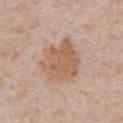{"lighting": "white-light", "lesion_size": {"long_diameter_mm_approx": 5.0}, "image": {"source": "total-body photography crop", "field_of_view_mm": 15}, "automated_metrics": {"area_mm2_approx": 18.0, "eccentricity": 0.5, "shape_asymmetry": 0.2, "cielab_L": 59, "cielab_a": 19, "cielab_b": 31, "vs_skin_darker_L": 9.0, "vs_skin_contrast_norm": 7.0}, "site": "abdomen", "patient": {"sex": "male", "age_approx": 65}}